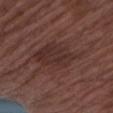This lesion was catalogued during total-body skin photography and was not selected for biopsy.
A male patient aged 73 to 77.
Captured under white-light illumination.
The lesion's longest dimension is about 7 mm.
A 15 mm close-up tile from a total-body photography series done for melanoma screening.
From the arm.